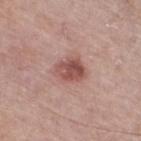The lesion was photographed on a routine skin check and not biopsied; there is no pathology result. The total-body-photography lesion software estimated a lesion color around L≈51 a*≈24 b*≈24 in CIELAB, a lesion–skin lightness drop of about 12, and a normalized border contrast of about 8.5. On the right thigh. The tile uses white-light illumination. A male subject, aged 73 to 77. Cropped from a total-body skin-imaging series; the visible field is about 15 mm.